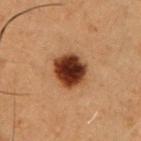Imaged during a routine full-body skin examination; the lesion was not biopsied and no histopathology is available. The subject is a male about 50 years old. Cropped from a total-body skin-imaging series; the visible field is about 15 mm. Measured at roughly 4.5 mm in maximum diameter. Captured under cross-polarized illumination. From the chest.